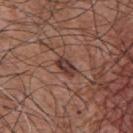follow-up=total-body-photography surveillance lesion; no biopsy
patient=male, about 55 years old
anatomic site=the chest
acquisition=total-body-photography crop, ~15 mm field of view
lighting=white-light illumination
size=~3 mm (longest diameter)